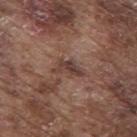{
  "biopsy_status": "not biopsied; imaged during a skin examination",
  "lesion_size": {
    "long_diameter_mm_approx": 3.5
  },
  "patient": {
    "sex": "male",
    "age_approx": 75
  },
  "site": "back",
  "image": {
    "source": "total-body photography crop",
    "field_of_view_mm": 15
  }
}